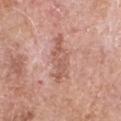{
  "biopsy_status": "not biopsied; imaged during a skin examination",
  "lesion_size": {
    "long_diameter_mm_approx": 6.0
  },
  "automated_metrics": {
    "cielab_L": 58,
    "cielab_a": 23,
    "cielab_b": 27,
    "vs_skin_darker_L": 9.0,
    "vs_skin_contrast_norm": 6.0
  },
  "image": {
    "source": "total-body photography crop",
    "field_of_view_mm": 15
  },
  "patient": {
    "sex": "male",
    "age_approx": 65
  },
  "site": "right upper arm",
  "lighting": "white-light"
}